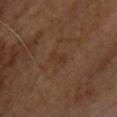– workup: total-body-photography surveillance lesion; no biopsy
– image-analysis metrics: an automated nevus-likeness rating near 0 out of 100
– site: the back
– subject: male, aged 58–62
– imaging modality: 15 mm crop, total-body photography
– lighting: cross-polarized illumination